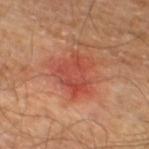Case summary:
- notes: imaged on a skin check; not biopsied
- illumination: cross-polarized
- acquisition: total-body-photography crop, ~15 mm field of view
- anatomic site: the left lower leg
- patient: male, aged around 55
- size: ≈4.5 mm